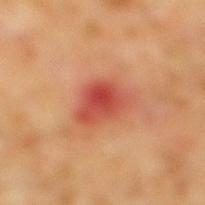Assessment:
Captured during whole-body skin photography for melanoma surveillance; the lesion was not biopsied.
Acquisition and patient details:
On the right lower leg. This image is a 15 mm lesion crop taken from a total-body photograph. Captured under cross-polarized illumination. The lesion-visualizer software estimated a footprint of about 12 mm² and an outline eccentricity of about 0.75 (0 = round, 1 = elongated). And it measured a mean CIELAB color near L≈49 a*≈33 b*≈33 and a normalized lesion–skin contrast near 8. The subject is a male about 60 years old. The lesion's longest dimension is about 5 mm.